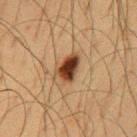Clinical impression: No biopsy was performed on this lesion — it was imaged during a full skin examination and was not determined to be concerning. Clinical summary: The lesion is located on the chest. Cropped from a whole-body photographic skin survey; the tile spans about 15 mm. The recorded lesion diameter is about 3.5 mm. A male subject, aged around 60. The tile uses cross-polarized illumination.